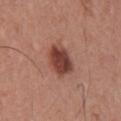biopsy status — catalogued during a skin exam; not biopsied
anatomic site — the chest
patient — male, about 40 years old
image source — total-body-photography crop, ~15 mm field of view
size — ~4.5 mm (longest diameter)
lighting — white-light